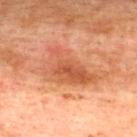Clinical impression: The lesion was tiled from a total-body skin photograph and was not biopsied. Image and clinical context: Cropped from a total-body skin-imaging series; the visible field is about 15 mm. Located on the upper back. A male subject aged approximately 75.